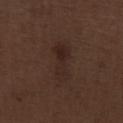follow-up=no biopsy performed (imaged during a skin exam) | lighting=white-light illumination | patient=male, approximately 70 years of age | size=~5.5 mm (longest diameter) | image source=~15 mm crop, total-body skin-cancer survey | body site=the leg.